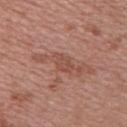The lesion was photographed on a routine skin check and not biopsied; there is no pathology result. Imaged with white-light lighting. A 15 mm crop from a total-body photograph taken for skin-cancer surveillance. Longest diameter approximately 3 mm. The lesion is on the upper back. A female subject, in their 50s.